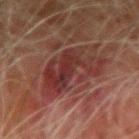patient: male, aged around 80
lesion size: ≈6 mm
lighting: cross-polarized
imaging modality: ~15 mm crop, total-body skin-cancer survey
site: the left forearm
automated lesion analysis: a footprint of about 18 mm², an outline eccentricity of about 0.6 (0 = round, 1 = elongated), and two-axis asymmetry of about 0.55; an average lesion color of about L≈25 a*≈20 b*≈18 (CIELAB), a lesion–skin lightness drop of about 7, and a lesion-to-skin contrast of about 7.5 (normalized; higher = more distinct); a border-irregularity rating of about 7.5/10, internal color variation of about 5 on a 0–10 scale, and radial color variation of about 1.5; an automated nevus-likeness rating near 0 out of 100 and a lesion-detection confidence of about 100/100